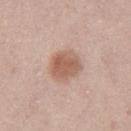Assessment: Imaged during a routine full-body skin examination; the lesion was not biopsied and no histopathology is available. Context: Measured at roughly 4 mm in maximum diameter. Located on the leg. Automated image analysis of the tile measured a lesion color around L≈59 a*≈20 b*≈28 in CIELAB, roughly 11 lightness units darker than nearby skin, and a normalized lesion–skin contrast near 7.5. Captured under white-light illumination. A female patient, approximately 20 years of age. A 15 mm crop from a total-body photograph taken for skin-cancer surveillance.